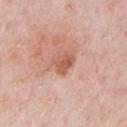Q: Was a biopsy performed?
A: total-body-photography surveillance lesion; no biopsy
Q: Lesion location?
A: the chest
Q: Patient demographics?
A: male, aged 58–62
Q: How was this image acquired?
A: ~15 mm crop, total-body skin-cancer survey